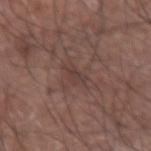This lesion was catalogued during total-body skin photography and was not selected for biopsy.
Located on the right forearm.
Imaged with white-light lighting.
Cropped from a whole-body photographic skin survey; the tile spans about 15 mm.
The patient is a male aged around 75.
Longest diameter approximately 3 mm.